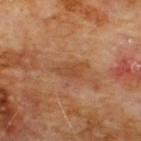{
  "biopsy_status": "not biopsied; imaged during a skin examination",
  "patient": {
    "sex": "male",
    "age_approx": 60
  },
  "lighting": "cross-polarized",
  "automated_metrics": {
    "area_mm2_approx": 5.0,
    "eccentricity": 0.85,
    "color_variation_0_10": 1.5,
    "peripheral_color_asymmetry": 0.5,
    "nevus_likeness_0_100": 0,
    "lesion_detection_confidence_0_100": 100
  },
  "site": "chest",
  "lesion_size": {
    "long_diameter_mm_approx": 4.0
  },
  "image": {
    "source": "total-body photography crop",
    "field_of_view_mm": 15
  }
}